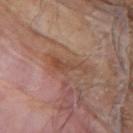notes: no biopsy performed (imaged during a skin exam)
automated metrics: a border-irregularity rating of about 7/10, a color-variation rating of about 2/10, and radial color variation of about 0; a nevus-likeness score of about 0/100 and a lesion-detection confidence of about 95/100
image source: ~15 mm tile from a whole-body skin photo
size: about 4.5 mm
lighting: white-light illumination
site: the upper back
subject: male, aged around 80